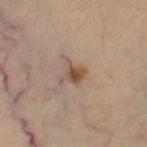{"biopsy_status": "not biopsied; imaged during a skin examination"}